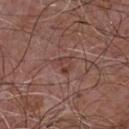<case>
<patient>
  <sex>male</sex>
  <age_approx>55</age_approx>
</patient>
<site>chest</site>
<image>
  <source>total-body photography crop</source>
  <field_of_view_mm>15</field_of_view_mm>
</image>
<lesion_size>
  <long_diameter_mm_approx>2.5</long_diameter_mm_approx>
</lesion_size>
<lighting>white-light</lighting>
<automated_metrics>
  <area_mm2_approx>3.5</area_mm2_approx>
  <eccentricity>0.65</eccentricity>
  <shape_asymmetry>0.4</shape_asymmetry>
  <vs_skin_darker_L>7.0</vs_skin_darker_L>
  <nevus_likeness_0_100>0</nevus_likeness_0_100>
</automated_metrics>
</case>